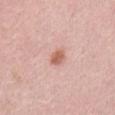Captured during whole-body skin photography for melanoma surveillance; the lesion was not biopsied.